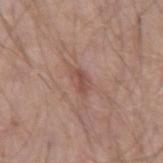Notes:
* notes · catalogued during a skin exam; not biopsied
* automated metrics · a footprint of about 3 mm², an eccentricity of roughly 0.85, and a shape-asymmetry score of about 0.35 (0 = symmetric); roughly 9 lightness units darker than nearby skin; a border-irregularity index near 4/10, a within-lesion color-variation index near 1/10, and radial color variation of about 0; a classifier nevus-likeness of about 0/100 and a lesion-detection confidence of about 100/100
* lesion diameter · ~2.5 mm (longest diameter)
* lighting · white-light
* location · the left forearm
* imaging modality · total-body-photography crop, ~15 mm field of view
* patient · male, aged 48–52